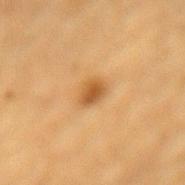Notes:
– notes · no biopsy performed (imaged during a skin exam)
– patient · male, in their mid-80s or older
– illumination · cross-polarized illumination
– location · the mid back
– image · total-body-photography crop, ~15 mm field of view